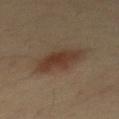follow-up: no biopsy performed (imaged during a skin exam).